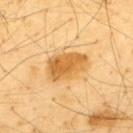notes = no biopsy performed (imaged during a skin exam); patient = male, about 65 years old; site = the upper back; automated lesion analysis = a border-irregularity rating of about 2/10 and a peripheral color-asymmetry measure near 1.5; illumination = cross-polarized illumination; lesion size = about 5 mm; image = ~15 mm crop, total-body skin-cancer survey.